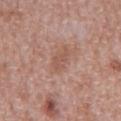Q: Is there a histopathology result?
A: catalogued during a skin exam; not biopsied
Q: What kind of image is this?
A: ~15 mm crop, total-body skin-cancer survey
Q: What is the anatomic site?
A: the abdomen
Q: What lighting was used for the tile?
A: white-light
Q: Patient demographics?
A: male, in their mid- to late 60s
Q: How large is the lesion?
A: about 3.5 mm